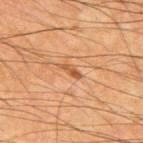Impression:
Captured during whole-body skin photography for melanoma surveillance; the lesion was not biopsied.
Acquisition and patient details:
From the upper back. The lesion's longest dimension is about 2.5 mm. A roughly 15 mm field-of-view crop from a total-body skin photograph. The tile uses cross-polarized illumination. The lesion-visualizer software estimated a footprint of about 2 mm² and an eccentricity of roughly 0.95. The analysis additionally found a border-irregularity rating of about 3.5/10, internal color variation of about 0 on a 0–10 scale, and peripheral color asymmetry of about 0. The subject is a male aged 63 to 67.